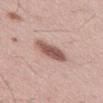Notes:
– biopsy status — catalogued during a skin exam; not biopsied
– image source — ~15 mm crop, total-body skin-cancer survey
– patient — male, aged 53–57
– image-analysis metrics — a lesion area of about 8.5 mm², an eccentricity of roughly 0.9, and a shape-asymmetry score of about 0.15 (0 = symmetric); a border-irregularity index near 2/10 and radial color variation of about 1; a nevus-likeness score of about 90/100 and a detector confidence of about 100 out of 100 that the crop contains a lesion
– diameter — ≈4.5 mm
– lighting — white-light illumination
– location — the front of the torso